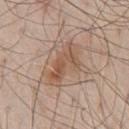Notes:
- biopsy status: total-body-photography surveillance lesion; no biopsy
- automated metrics: a border-irregularity rating of about 4.5/10 and internal color variation of about 5 on a 0–10 scale; an automated nevus-likeness rating near 55 out of 100 and a detector confidence of about 100 out of 100 that the crop contains a lesion
- lesion size: ~6 mm (longest diameter)
- subject: male, aged 78–82
- image: ~15 mm tile from a whole-body skin photo
- anatomic site: the chest